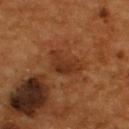Impression:
Part of a total-body skin-imaging series; this lesion was reviewed on a skin check and was not flagged for biopsy.
Background:
A male patient, approximately 55 years of age. The lesion's longest dimension is about 4 mm. From the back. The tile uses cross-polarized illumination. Automated image analysis of the tile measured a lesion color around L≈31 a*≈22 b*≈30 in CIELAB, a lesion–skin lightness drop of about 7, and a normalized lesion–skin contrast near 7. And it measured a nevus-likeness score of about 0/100. Cropped from a total-body skin-imaging series; the visible field is about 15 mm.